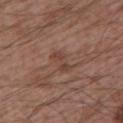Notes:
– workup — no biopsy performed (imaged during a skin exam)
– location — the left upper arm
– subject — male, about 65 years old
– image-analysis metrics — a lesion area of about 4.5 mm², a shape eccentricity near 0.85, and a shape-asymmetry score of about 0.3 (0 = symmetric); a lesion–skin lightness drop of about 7 and a normalized border contrast of about 6
– tile lighting — white-light
– image — 15 mm crop, total-body photography